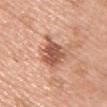Cropped from a whole-body photographic skin survey; the tile spans about 15 mm. A female patient, about 60 years old. Captured under white-light illumination. An algorithmic analysis of the crop reported an average lesion color of about L≈55 a*≈24 b*≈30 (CIELAB), roughly 15 lightness units darker than nearby skin, and a normalized border contrast of about 9.5. The software also gave border irregularity of about 4.5 on a 0–10 scale, a within-lesion color-variation index near 3.5/10, and a peripheral color-asymmetry measure near 1. The analysis additionally found a detector confidence of about 100 out of 100 that the crop contains a lesion. Located on the front of the torso.